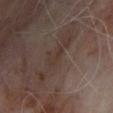The lesion was tiled from a total-body skin photograph and was not biopsied. The patient is a male aged around 65. The lesion is located on the chest. This image is a 15 mm lesion crop taken from a total-body photograph.